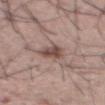Background: A 15 mm close-up extracted from a 3D total-body photography capture. A male subject aged 53 to 57. The lesion is located on the abdomen.